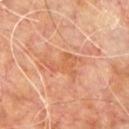Assessment: The lesion was photographed on a routine skin check and not biopsied; there is no pathology result. Clinical summary: The lesion is on the upper back. Approximately 5 mm at its widest. A male patient in their 60s. This image is a 15 mm lesion crop taken from a total-body photograph.